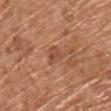Impression:
Part of a total-body skin-imaging series; this lesion was reviewed on a skin check and was not flagged for biopsy.
Acquisition and patient details:
Located on the upper back. About 3 mm across. Imaged with white-light lighting. A region of skin cropped from a whole-body photographic capture, roughly 15 mm wide. A male subject aged around 70.